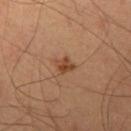This lesion was catalogued during total-body skin photography and was not selected for biopsy. A close-up tile cropped from a whole-body skin photograph, about 15 mm across. A male patient, aged 58 to 62. From the leg. This is a cross-polarized tile. Approximately 2 mm at its widest.